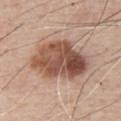  biopsy_status: not biopsied; imaged during a skin examination
  image:
    source: total-body photography crop
    field_of_view_mm: 15
  patient:
    sex: male
    age_approx: 55
  site: chest